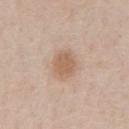The lesion was photographed on a routine skin check and not biopsied; there is no pathology result. A region of skin cropped from a whole-body photographic capture, roughly 15 mm wide. A male patient aged approximately 60. Measured at roughly 3 mm in maximum diameter. Located on the chest.